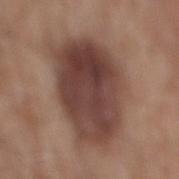Case summary:
– biopsy status — catalogued during a skin exam; not biopsied
– automated metrics — a border-irregularity index near 2.5/10, a color-variation rating of about 6/10, and a peripheral color-asymmetry measure near 2
– diameter — about 9 mm
– acquisition — total-body-photography crop, ~15 mm field of view
– subject — male, aged approximately 60
– location — the mid back
– illumination — white-light illumination